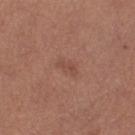No biopsy was performed on this lesion — it was imaged during a full skin examination and was not determined to be concerning. The recorded lesion diameter is about 2.5 mm. A region of skin cropped from a whole-body photographic capture, roughly 15 mm wide. A female subject, aged 53–57. Captured under white-light illumination. From the right thigh. Automated image analysis of the tile measured a border-irregularity index near 3/10, internal color variation of about 0.5 on a 0–10 scale, and peripheral color asymmetry of about 0. The software also gave an automated nevus-likeness rating near 0 out of 100 and lesion-presence confidence of about 100/100.